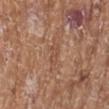Case summary:
– workup · catalogued during a skin exam; not biopsied
– body site · the left lower leg
– automated metrics · a footprint of about 3.5 mm², a shape eccentricity near 0.85, and a shape-asymmetry score of about 0.2 (0 = symmetric); a mean CIELAB color near L≈50 a*≈21 b*≈30 and a normalized lesion–skin contrast near 4.5
– imaging modality · 15 mm crop, total-body photography
– patient · female, aged approximately 75